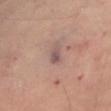Findings:
- acquisition — ~15 mm tile from a whole-body skin photo
- site — the leg
- subject — female, aged 58 to 62